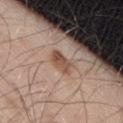On the right thigh. The subject is a male roughly 30 years of age. A 15 mm close-up tile from a total-body photography series done for melanoma screening.